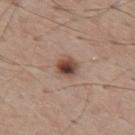Clinical impression: Part of a total-body skin-imaging series; this lesion was reviewed on a skin check and was not flagged for biopsy. Acquisition and patient details: Automated tile analysis of the lesion measured an area of roughly 5 mm², a shape eccentricity near 0.4, and a symmetry-axis asymmetry near 0.2. The software also gave a lesion–skin lightness drop of about 15. And it measured a border-irregularity index near 1.5/10, a color-variation rating of about 8.5/10, and a peripheral color-asymmetry measure near 2.5. The software also gave a detector confidence of about 100 out of 100 that the crop contains a lesion. Located on the upper back. A close-up tile cropped from a whole-body skin photograph, about 15 mm across. A male patient approximately 60 years of age.